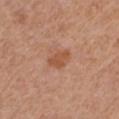biopsy status: no biopsy performed (imaged during a skin exam).